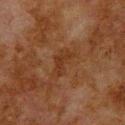follow-up = no biopsy performed (imaged during a skin exam); subject = male, aged 78–82; lighting = cross-polarized; diameter = ≈3 mm; image = ~15 mm crop, total-body skin-cancer survey; body site = the upper back.